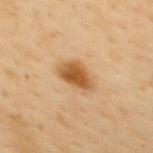The lesion was photographed on a routine skin check and not biopsied; there is no pathology result.
Automated tile analysis of the lesion measured a border-irregularity rating of about 2.5/10, a color-variation rating of about 4/10, and radial color variation of about 1. It also reported a classifier nevus-likeness of about 95/100 and a lesion-detection confidence of about 100/100.
From the upper back.
Longest diameter approximately 4 mm.
The tile uses cross-polarized illumination.
A 15 mm crop from a total-body photograph taken for skin-cancer surveillance.
A female patient approximately 50 years of age.